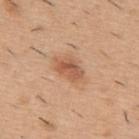| key | value |
|---|---|
| follow-up | no biopsy performed (imaged during a skin exam) |
| TBP lesion metrics | a footprint of about 6 mm² and a shape eccentricity near 0.8; a lesion color around L≈56 a*≈23 b*≈34 in CIELAB, roughly 11 lightness units darker than nearby skin, and a lesion-to-skin contrast of about 7 (normalized; higher = more distinct); a border-irregularity index near 2.5/10, a color-variation rating of about 4/10, and radial color variation of about 1.5 |
| illumination | white-light |
| size | ≈3.5 mm |
| patient | male, about 40 years old |
| image source | 15 mm crop, total-body photography |
| body site | the upper back |